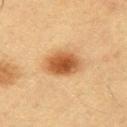follow-up: imaged on a skin check; not biopsied
patient: male, aged around 55
lesion diameter: ~4 mm (longest diameter)
automated lesion analysis: roughly 14 lightness units darker than nearby skin; a border-irregularity rating of about 1.5/10 and internal color variation of about 5 on a 0–10 scale; an automated nevus-likeness rating near 100 out of 100 and lesion-presence confidence of about 100/100
imaging modality: ~15 mm tile from a whole-body skin photo
site: the left upper arm